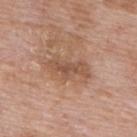Imaged during a routine full-body skin examination; the lesion was not biopsied and no histopathology is available. Located on the upper back. A male subject, aged 58 to 62. A region of skin cropped from a whole-body photographic capture, roughly 15 mm wide.